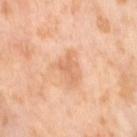| field | value |
|---|---|
| workup | no biopsy performed (imaged during a skin exam) |
| lesion diameter | ~3 mm (longest diameter) |
| acquisition | ~15 mm crop, total-body skin-cancer survey |
| automated metrics | lesion-presence confidence of about 100/100 |
| subject | female, roughly 55 years of age |
| body site | the right thigh |
| tile lighting | cross-polarized illumination |